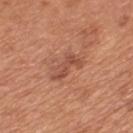Recorded during total-body skin imaging; not selected for excision or biopsy. A lesion tile, about 15 mm wide, cut from a 3D total-body photograph. Measured at roughly 4 mm in maximum diameter. A male subject aged 63–67. This is a white-light tile. From the mid back. Automated tile analysis of the lesion measured border irregularity of about 5.5 on a 0–10 scale, a color-variation rating of about 2.5/10, and a peripheral color-asymmetry measure near 1. And it measured an automated nevus-likeness rating near 5 out of 100 and a detector confidence of about 100 out of 100 that the crop contains a lesion.